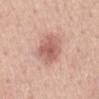– anatomic site: the mid back
– subject: male, about 55 years old
– illumination: white-light
– diameter: ~5 mm (longest diameter)
– imaging modality: ~15 mm tile from a whole-body skin photo